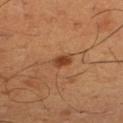The lesion was photographed on a routine skin check and not biopsied; there is no pathology result.
Located on the right thigh.
A male subject aged 48–52.
The tile uses cross-polarized illumination.
About 2.5 mm across.
Automated image analysis of the tile measured an area of roughly 3.5 mm² and two-axis asymmetry of about 0.25. The analysis additionally found a border-irregularity rating of about 2.5/10, a within-lesion color-variation index near 2/10, and radial color variation of about 0.5.
A lesion tile, about 15 mm wide, cut from a 3D total-body photograph.